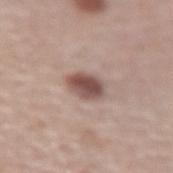Impression:
No biopsy was performed on this lesion — it was imaged during a full skin examination and was not determined to be concerning.
Context:
Approximately 3.5 mm at its widest. The patient is a female approximately 60 years of age. The lesion is located on the left forearm. Cropped from a whole-body photographic skin survey; the tile spans about 15 mm. The tile uses white-light illumination.